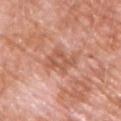<case>
<biopsy_status>not biopsied; imaged during a skin examination</biopsy_status>
<patient>
  <sex>male</sex>
  <age_approx>60</age_approx>
</patient>
<site>chest</site>
<lesion_size>
  <long_diameter_mm_approx>4.5</long_diameter_mm_approx>
</lesion_size>
<image>
  <source>total-body photography crop</source>
  <field_of_view_mm>15</field_of_view_mm>
</image>
<lighting>white-light</lighting>
<automated_metrics>
  <area_mm2_approx>8.5</area_mm2_approx>
  <eccentricity>0.85</eccentricity>
  <shape_asymmetry>0.35</shape_asymmetry>
  <cielab_L>57</cielab_L>
  <cielab_a>26</cielab_a>
  <cielab_b>32</cielab_b>
  <vs_skin_darker_L>9.0</vs_skin_darker_L>
  <vs_skin_contrast_norm>6.5</vs_skin_contrast_norm>
  <color_variation_0_10>4.0</color_variation_0_10>
</automated_metrics>
</case>